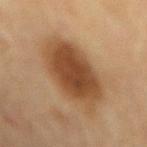workup: no biopsy performed (imaged during a skin exam); lighting: cross-polarized; site: the back; acquisition: ~15 mm tile from a whole-body skin photo; lesion size: about 8.5 mm; subject: female, roughly 80 years of age.